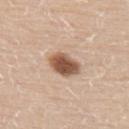- patient — male, roughly 60 years of age
- tile lighting — white-light illumination
- imaging modality — total-body-photography crop, ~15 mm field of view
- location — the upper back